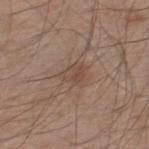  site: leg
  lighting: white-light
  patient:
    sex: male
    age_approx: 60
  automated_metrics:
    area_mm2_approx: 5.5
    shape_asymmetry: 0.35
    nevus_likeness_0_100: 5
  image:
    source: total-body photography crop
    field_of_view_mm: 15